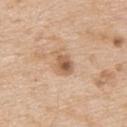Impression:
Recorded during total-body skin imaging; not selected for excision or biopsy.
Clinical summary:
The tile uses white-light illumination. Cropped from a total-body skin-imaging series; the visible field is about 15 mm. The lesion is on the upper back. The recorded lesion diameter is about 3 mm. The total-body-photography lesion software estimated a shape eccentricity near 0.7 and a symmetry-axis asymmetry near 0.3. It also reported a normalized border contrast of about 7.5. The software also gave a color-variation rating of about 7/10 and radial color variation of about 2.5. A male subject approximately 65 years of age.